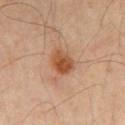Assessment: This lesion was catalogued during total-body skin photography and was not selected for biopsy.